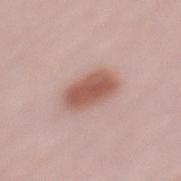Captured during whole-body skin photography for melanoma surveillance; the lesion was not biopsied.
This is a white-light tile.
The lesion-visualizer software estimated a footprint of about 12 mm², a shape eccentricity near 0.8, and a shape-asymmetry score of about 0.1 (0 = symmetric). It also reported a lesion color around L≈56 a*≈23 b*≈26 in CIELAB and a normalized lesion–skin contrast near 9.
On the mid back.
A close-up tile cropped from a whole-body skin photograph, about 15 mm across.
The subject is a female aged around 40.